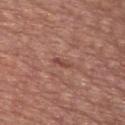Q: Is there a histopathology result?
A: catalogued during a skin exam; not biopsied
Q: Automated lesion metrics?
A: about 9 CIELAB-L* units darker than the surrounding skin and a normalized border contrast of about 6.5; border irregularity of about 3.5 on a 0–10 scale, internal color variation of about 0 on a 0–10 scale, and radial color variation of about 0
Q: Who is the patient?
A: male, about 55 years old
Q: Lesion location?
A: the chest
Q: How was the tile lit?
A: white-light
Q: Lesion size?
A: about 2.5 mm
Q: What kind of image is this?
A: ~15 mm crop, total-body skin-cancer survey